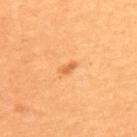<tbp_lesion>
  <image>
    <source>total-body photography crop</source>
    <field_of_view_mm>15</field_of_view_mm>
  </image>
  <patient>
    <sex>female</sex>
    <age_approx>35</age_approx>
  </patient>
  <lesion_size>
    <long_diameter_mm_approx>2.5</long_diameter_mm_approx>
  </lesion_size>
  <site>upper back</site>
</tbp_lesion>